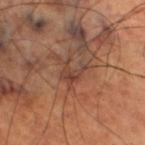Q: Was a biopsy performed?
A: no biopsy performed (imaged during a skin exam)
Q: Where on the body is the lesion?
A: the right thigh
Q: What is the lesion's diameter?
A: about 3 mm
Q: What are the patient's age and sex?
A: male, in their 70s
Q: What is the imaging modality?
A: total-body-photography crop, ~15 mm field of view
Q: How was the tile lit?
A: cross-polarized